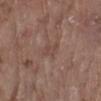No biopsy was performed on this lesion — it was imaged during a full skin examination and was not determined to be concerning. A female patient, aged 83 or older. The recorded lesion diameter is about 2.5 mm. Cropped from a whole-body photographic skin survey; the tile spans about 15 mm. From the left forearm.